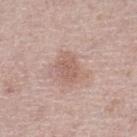Case summary:
* biopsy status · catalogued during a skin exam; not biopsied
* acquisition · total-body-photography crop, ~15 mm field of view
* subject · male, aged around 65
* location · the left lower leg
* TBP lesion metrics · a footprint of about 6.5 mm² and two-axis asymmetry of about 0.3